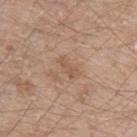Captured during whole-body skin photography for melanoma surveillance; the lesion was not biopsied. A 15 mm close-up extracted from a 3D total-body photography capture. On the arm. A male subject, in their 60s.